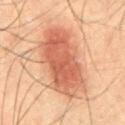Clinical impression: This lesion was catalogued during total-body skin photography and was not selected for biopsy. Background: Automated tile analysis of the lesion measured a lesion area of about 32 mm² and a symmetry-axis asymmetry near 0.15. The subject is a male in their 50s. A close-up tile cropped from a whole-body skin photograph, about 15 mm across. Imaged with cross-polarized lighting. The lesion is on the abdomen. Longest diameter approximately 9 mm.